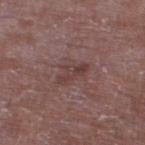notes: no biopsy performed (imaged during a skin exam) | site: the right thigh | imaging modality: ~15 mm tile from a whole-body skin photo | subject: male, aged approximately 65 | automated metrics: an area of roughly 5.5 mm², a shape eccentricity near 0.9, and a shape-asymmetry score of about 0.35 (0 = symmetric); a lesion–skin lightness drop of about 7 and a normalized lesion–skin contrast near 6 | lesion diameter: about 4 mm | tile lighting: white-light illumination.